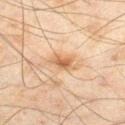No biopsy was performed on this lesion — it was imaged during a full skin examination and was not determined to be concerning. Imaged with cross-polarized lighting. A male subject, aged approximately 45. The lesion's longest dimension is about 3 mm. Automated image analysis of the tile measured an area of roughly 5.5 mm², a shape eccentricity near 0.7, and two-axis asymmetry of about 0.3. The software also gave an average lesion color of about L≈52 a*≈17 b*≈32 (CIELAB), a lesion–skin lightness drop of about 9, and a normalized lesion–skin contrast near 7. This image is a 15 mm lesion crop taken from a total-body photograph. On the right thigh.